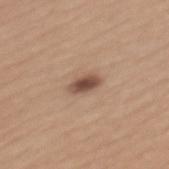follow-up = total-body-photography surveillance lesion; no biopsy | body site = the mid back | patient = female, approximately 45 years of age | diameter = ~3 mm (longest diameter) | imaging modality = 15 mm crop, total-body photography.